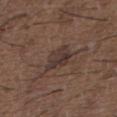workup: total-body-photography surveillance lesion; no biopsy | image: 15 mm crop, total-body photography | subject: male, approximately 50 years of age | lighting: white-light illumination | body site: the upper back | image-analysis metrics: a mean CIELAB color near L≈34 a*≈14 b*≈19 and roughly 8 lightness units darker than nearby skin; a classifier nevus-likeness of about 0/100 and a lesion-detection confidence of about 95/100.